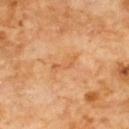Findings:
• biopsy status · no biopsy performed (imaged during a skin exam)
• tile lighting · cross-polarized
• body site · the front of the torso
• image source · ~15 mm tile from a whole-body skin photo
• diameter · ~3 mm (longest diameter)
• automated lesion analysis · a lesion area of about 3 mm², an outline eccentricity of about 0.95 (0 = round, 1 = elongated), and two-axis asymmetry of about 0.55; a lesion-detection confidence of about 100/100
• patient · male, aged around 60